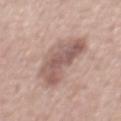workup=no biopsy performed (imaged during a skin exam) | patient=male, aged approximately 50 | location=the abdomen | imaging modality=15 mm crop, total-body photography.